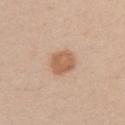Context: A lesion tile, about 15 mm wide, cut from a 3D total-body photograph. A female subject roughly 25 years of age. The lesion is located on the right upper arm.